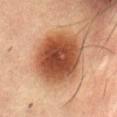Impression:
Captured during whole-body skin photography for melanoma surveillance; the lesion was not biopsied.
Background:
Imaged with cross-polarized lighting. On the mid back. A roughly 15 mm field-of-view crop from a total-body skin photograph. The subject is a male aged around 55. The recorded lesion diameter is about 6.5 mm.